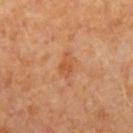Recorded during total-body skin imaging; not selected for excision or biopsy. A female subject aged around 50. A 15 mm close-up extracted from a 3D total-body photography capture. This is a cross-polarized tile. On the right forearm. The lesion's longest dimension is about 3 mm.